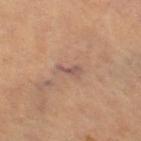Clinical impression: This lesion was catalogued during total-body skin photography and was not selected for biopsy. Clinical summary: The total-body-photography lesion software estimated a footprint of about 3 mm² and a symmetry-axis asymmetry near 0.45. The software also gave a mean CIELAB color near L≈53 a*≈19 b*≈25, a lesion–skin lightness drop of about 8, and a normalized border contrast of about 7. It also reported a border-irregularity index near 5/10 and internal color variation of about 0.5 on a 0–10 scale. A subject aged 58 to 62. Longest diameter approximately 3 mm. Captured under cross-polarized illumination. This image is a 15 mm lesion crop taken from a total-body photograph. Located on the left thigh.